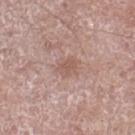The lesion was tiled from a total-body skin photograph and was not biopsied.
A 15 mm close-up tile from a total-body photography series done for melanoma screening.
Located on the right thigh.
A female patient, aged around 75.